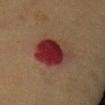{"biopsy_status": "not biopsied; imaged during a skin examination", "patient": {"sex": "female", "age_approx": 40}, "lighting": "cross-polarized", "image": {"source": "total-body photography crop", "field_of_view_mm": 15}, "site": "abdomen", "lesion_size": {"long_diameter_mm_approx": 5.5}, "automated_metrics": {"area_mm2_approx": 17.0, "shape_asymmetry": 0.15, "cielab_L": 27, "cielab_a": 26, "cielab_b": 22, "vs_skin_contrast_norm": 12.5, "border_irregularity_0_10": 1.5, "color_variation_0_10": 9.5, "peripheral_color_asymmetry": 3.5, "nevus_likeness_0_100": 0, "lesion_detection_confidence_0_100": 100}}